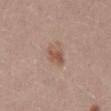No biopsy was performed on this lesion — it was imaged during a full skin examination and was not determined to be concerning.
On the abdomen.
A close-up tile cropped from a whole-body skin photograph, about 15 mm across.
About 2.5 mm across.
A female patient approximately 40 years of age.
The total-body-photography lesion software estimated an average lesion color of about L≈54 a*≈18 b*≈28 (CIELAB), a lesion–skin lightness drop of about 9, and a lesion-to-skin contrast of about 7 (normalized; higher = more distinct). And it measured border irregularity of about 1.5 on a 0–10 scale, internal color variation of about 3.5 on a 0–10 scale, and a peripheral color-asymmetry measure near 1.
Captured under white-light illumination.